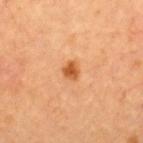| feature | finding |
|---|---|
| follow-up | imaged on a skin check; not biopsied |
| diameter | ~2.5 mm (longest diameter) |
| patient | female, in their 70s |
| lighting | cross-polarized illumination |
| acquisition | ~15 mm crop, total-body skin-cancer survey |
| anatomic site | the upper back |